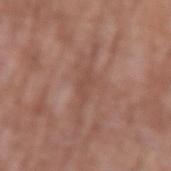The total-body-photography lesion software estimated a classifier nevus-likeness of about 0/100. This image is a 15 mm lesion crop taken from a total-body photograph. The patient is a male about 60 years old. The lesion's longest dimension is about 2.5 mm. From the left upper arm. The tile uses white-light illumination.